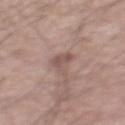Q: How large is the lesion?
A: ≈4 mm
Q: Who is the patient?
A: male, about 55 years old
Q: Lesion location?
A: the mid back
Q: What kind of image is this?
A: total-body-photography crop, ~15 mm field of view
Q: Illumination type?
A: white-light illumination